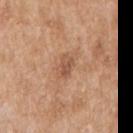image-analysis metrics: a nevus-likeness score of about 0/100 and a detector confidence of about 100 out of 100 that the crop contains a lesion; anatomic site: the right upper arm; patient: male, aged approximately 60; image: ~15 mm tile from a whole-body skin photo; size: ~2.5 mm (longest diameter); illumination: white-light illumination.